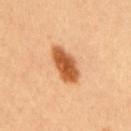Imaged during a routine full-body skin examination; the lesion was not biopsied and no histopathology is available.
The lesion's longest dimension is about 5 mm.
A 15 mm crop from a total-body photograph taken for skin-cancer surveillance.
The subject is a female approximately 50 years of age.